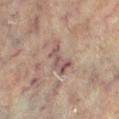follow-up: no biopsy performed (imaged during a skin exam)
illumination: cross-polarized
image: 15 mm crop, total-body photography
location: the leg
patient: female, in their 80s